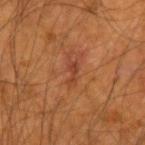  biopsy_status: not biopsied; imaged during a skin examination
  site: left forearm
  patient:
    sex: male
    age_approx: 55
  automated_metrics:
    area_mm2_approx: 3.0
    eccentricity: 0.9
    shape_asymmetry: 0.45
    lesion_detection_confidence_0_100: 100
  lighting: cross-polarized
  image:
    source: total-body photography crop
    field_of_view_mm: 15